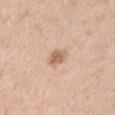No biopsy was performed on this lesion — it was imaged during a full skin examination and was not determined to be concerning. The lesion is on the right upper arm. A 15 mm close-up tile from a total-body photography series done for melanoma screening. An algorithmic analysis of the crop reported an automated nevus-likeness rating near 55 out of 100 and a lesion-detection confidence of about 100/100. A female patient roughly 40 years of age.